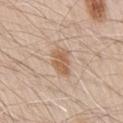The lesion was tiled from a total-body skin photograph and was not biopsied. The lesion-visualizer software estimated border irregularity of about 3 on a 0–10 scale, internal color variation of about 2.5 on a 0–10 scale, and peripheral color asymmetry of about 1. It also reported an automated nevus-likeness rating near 90 out of 100 and a detector confidence of about 100 out of 100 that the crop contains a lesion. A region of skin cropped from a whole-body photographic capture, roughly 15 mm wide. A male subject about 70 years old. From the right upper arm.